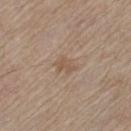This lesion was catalogued during total-body skin photography and was not selected for biopsy. A 15 mm crop from a total-body photograph taken for skin-cancer surveillance. The lesion is on the left thigh. The patient is a female in their mid-60s.